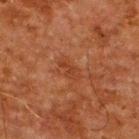• follow-up — no biopsy performed (imaged during a skin exam)
• site — the upper back
• automated lesion analysis — a lesion color around L≈31 a*≈22 b*≈29 in CIELAB, a lesion–skin lightness drop of about 5, and a lesion-to-skin contrast of about 5.5 (normalized; higher = more distinct); a border-irregularity rating of about 4/10 and peripheral color asymmetry of about 0; a classifier nevus-likeness of about 0/100
• subject — male, aged 58–62
• image source — ~15 mm tile from a whole-body skin photo
• lesion size — about 3 mm